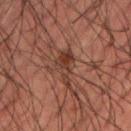Recorded during total-body skin imaging; not selected for excision or biopsy. A male patient, roughly 60 years of age. The lesion-visualizer software estimated a lesion color around L≈33 a*≈19 b*≈24 in CIELAB, about 8 CIELAB-L* units darker than the surrounding skin, and a lesion-to-skin contrast of about 8 (normalized; higher = more distinct). The software also gave border irregularity of about 5.5 on a 0–10 scale, internal color variation of about 4.5 on a 0–10 scale, and a peripheral color-asymmetry measure near 1.5. It also reported lesion-presence confidence of about 65/100. This image is a 15 mm lesion crop taken from a total-body photograph. The recorded lesion diameter is about 5 mm.